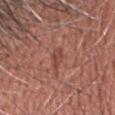  biopsy_status: not biopsied; imaged during a skin examination
  lighting: white-light
  lesion_size:
    long_diameter_mm_approx: 3.0
  patient:
    sex: male
    age_approx: 65
  site: head or neck
  image:
    source: total-body photography crop
    field_of_view_mm: 15